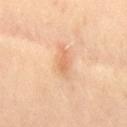The lesion was photographed on a routine skin check and not biopsied; there is no pathology result. A male patient aged around 55. A 15 mm crop from a total-body photograph taken for skin-cancer surveillance. About 3 mm across. The tile uses cross-polarized illumination. An algorithmic analysis of the crop reported a footprint of about 3 mm², an outline eccentricity of about 0.85 (0 = round, 1 = elongated), and a symmetry-axis asymmetry near 0.25. The software also gave roughly 8 lightness units darker than nearby skin and a normalized lesion–skin contrast near 5.5. The lesion is located on the right thigh.